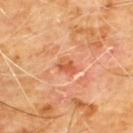Imaged during a routine full-body skin examination; the lesion was not biopsied and no histopathology is available.
On the chest.
A roughly 15 mm field-of-view crop from a total-body skin photograph.
A male patient roughly 60 years of age.
Longest diameter approximately 2.5 mm.
The lesion-visualizer software estimated an area of roughly 3.5 mm², a shape eccentricity near 0.7, and a symmetry-axis asymmetry near 0.3. And it measured a border-irregularity rating of about 3/10 and peripheral color asymmetry of about 1.5.